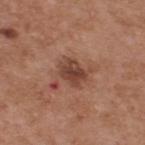Longest diameter approximately 3.5 mm. This is a white-light tile. The patient is a male approximately 55 years of age. From the upper back. Automated tile analysis of the lesion measured a mean CIELAB color near L≈43 a*≈22 b*≈28 and a normalized border contrast of about 8.5. And it measured a border-irregularity rating of about 2/10, a color-variation rating of about 3.5/10, and peripheral color asymmetry of about 1. A 15 mm close-up tile from a total-body photography series done for melanoma screening.